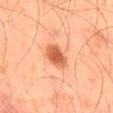Case summary:
- workup — catalogued during a skin exam; not biopsied
- patient — male, aged 43 to 47
- automated metrics — a footprint of about 6 mm² and a shape-asymmetry score of about 0.15 (0 = symmetric); a border-irregularity rating of about 1.5/10, a color-variation rating of about 2.5/10, and peripheral color asymmetry of about 0.5
- diameter — ~3 mm (longest diameter)
- location — the mid back
- imaging modality — 15 mm crop, total-body photography